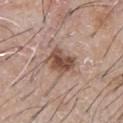{"biopsy_status": "not biopsied; imaged during a skin examination", "image": {"source": "total-body photography crop", "field_of_view_mm": 15}, "site": "chest", "lesion_size": {"long_diameter_mm_approx": 4.0}, "patient": {"sex": "male", "age_approx": 65}}